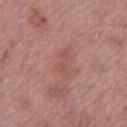| field | value |
|---|---|
| biopsy status | total-body-photography surveillance lesion; no biopsy |
| TBP lesion metrics | a footprint of about 3 mm², a shape eccentricity near 0.9, and a shape-asymmetry score of about 0.55 (0 = symmetric); border irregularity of about 7 on a 0–10 scale and internal color variation of about 0 on a 0–10 scale; a detector confidence of about 100 out of 100 that the crop contains a lesion |
| lesion size | ≈3 mm |
| image source | 15 mm crop, total-body photography |
| anatomic site | the right thigh |
| illumination | white-light |
| subject | male, about 55 years old |